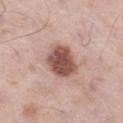{
  "biopsy_status": "not biopsied; imaged during a skin examination",
  "image": {
    "source": "total-body photography crop",
    "field_of_view_mm": 15
  },
  "patient": {
    "sex": "male",
    "age_approx": 55
  },
  "site": "right thigh"
}